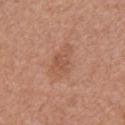Acquisition and patient details:
A 15 mm close-up tile from a total-body photography series done for melanoma screening. Located on the back. A female patient, aged 33 to 37. Captured under white-light illumination. Measured at roughly 4.5 mm in maximum diameter.